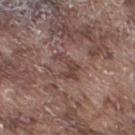Q: Was a biopsy performed?
A: total-body-photography surveillance lesion; no biopsy
Q: How was this image acquired?
A: total-body-photography crop, ~15 mm field of view
Q: What are the patient's age and sex?
A: male, approximately 75 years of age
Q: Where on the body is the lesion?
A: the right thigh